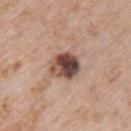Acquisition and patient details:
Measured at roughly 4 mm in maximum diameter. The lesion is on the left upper arm. A female patient, aged around 70. Cropped from a total-body skin-imaging series; the visible field is about 15 mm.